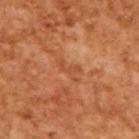Q: Was a biopsy performed?
A: total-body-photography surveillance lesion; no biopsy
Q: Lesion size?
A: about 3.5 mm
Q: What is the imaging modality?
A: ~15 mm crop, total-body skin-cancer survey
Q: Who is the patient?
A: male, aged 63–67
Q: Illumination type?
A: cross-polarized illumination